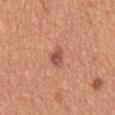The lesion was tiled from a total-body skin photograph and was not biopsied. The subject is a male aged 53–57. Measured at roughly 3 mm in maximum diameter. A region of skin cropped from a whole-body photographic capture, roughly 15 mm wide. On the mid back. Automated image analysis of the tile measured a mean CIELAB color near L≈53 a*≈25 b*≈30 and about 11 CIELAB-L* units darker than the surrounding skin. And it measured a within-lesion color-variation index near 3/10. It also reported an automated nevus-likeness rating near 70 out of 100 and a detector confidence of about 100 out of 100 that the crop contains a lesion. The tile uses white-light illumination.